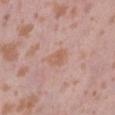Part of a total-body skin-imaging series; this lesion was reviewed on a skin check and was not flagged for biopsy. A 15 mm crop from a total-body photograph taken for skin-cancer surveillance. A female subject, aged 38 to 42. Captured under white-light illumination. On the abdomen. An algorithmic analysis of the crop reported an average lesion color of about L≈59 a*≈21 b*≈29 (CIELAB) and a normalized border contrast of about 6.5. The recorded lesion diameter is about 2.5 mm.